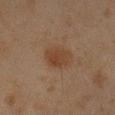workup — no biopsy performed (imaged during a skin exam) | size — ≈3.5 mm | imaging modality — 15 mm crop, total-body photography | patient — male, aged 43 to 47 | body site — the left forearm.